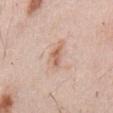This lesion was catalogued during total-body skin photography and was not selected for biopsy. The lesion's longest dimension is about 3 mm. Captured under white-light illumination. A close-up tile cropped from a whole-body skin photograph, about 15 mm across. A male patient, roughly 55 years of age. The lesion is on the abdomen.